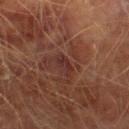The recorded lesion diameter is about 3 mm. A male patient, aged 73 to 77. A 15 mm close-up extracted from a 3D total-body photography capture. The total-body-photography lesion software estimated an eccentricity of roughly 0.95 and a symmetry-axis asymmetry near 0.3. On the right lower leg. This is a cross-polarized tile.